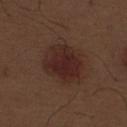Recorded during total-body skin imaging; not selected for excision or biopsy. The tile uses white-light illumination. A 15 mm crop from a total-body photograph taken for skin-cancer surveillance. Located on the abdomen. A male subject, about 70 years old.